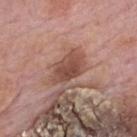  biopsy_status: not biopsied; imaged during a skin examination
  patient:
    sex: male
    age_approx: 75
  site: mid back
  image:
    source: total-body photography crop
    field_of_view_mm: 15